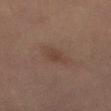Notes:
– workup: total-body-photography surveillance lesion; no biopsy
– subject: male, aged 43 to 47
– size: ≈3.5 mm
– illumination: cross-polarized
– imaging modality: 15 mm crop, total-body photography
– body site: the mid back
– automated lesion analysis: a lesion color around L≈32 a*≈14 b*≈20 in CIELAB and a normalized lesion–skin contrast near 5; internal color variation of about 2 on a 0–10 scale and radial color variation of about 0.5; a nevus-likeness score of about 30/100 and a detector confidence of about 100 out of 100 that the crop contains a lesion